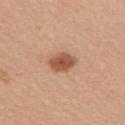notes: imaged on a skin check; not biopsied | patient: female, roughly 45 years of age | image source: ~15 mm crop, total-body skin-cancer survey | location: the right upper arm | lesion diameter: about 3 mm | lighting: white-light illumination.